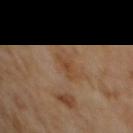Q: Was this lesion biopsied?
A: total-body-photography surveillance lesion; no biopsy
Q: Lesion size?
A: ~3 mm (longest diameter)
Q: What kind of image is this?
A: 15 mm crop, total-body photography
Q: Illumination type?
A: cross-polarized illumination
Q: Lesion location?
A: the chest
Q: What did automated image analysis measure?
A: an area of roughly 3 mm², an eccentricity of roughly 0.85, and a shape-asymmetry score of about 0.45 (0 = symmetric); a lesion color around L≈45 a*≈19 b*≈33 in CIELAB, a lesion–skin lightness drop of about 7, and a lesion-to-skin contrast of about 6 (normalized; higher = more distinct); an automated nevus-likeness rating near 0 out of 100
Q: What are the patient's age and sex?
A: female